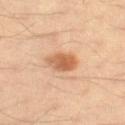biopsy status: no biopsy performed (imaged during a skin exam) | tile lighting: cross-polarized illumination | size: about 3.5 mm | acquisition: ~15 mm tile from a whole-body skin photo | location: the right thigh | automated metrics: an average lesion color of about L≈61 a*≈24 b*≈37 (CIELAB), roughly 13 lightness units darker than nearby skin, and a normalized border contrast of about 8.5; border irregularity of about 1 on a 0–10 scale; a nevus-likeness score of about 95/100 and a detector confidence of about 100 out of 100 that the crop contains a lesion | patient: male, aged 38 to 42.